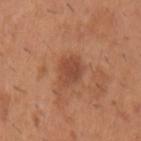Captured during whole-body skin photography for melanoma surveillance; the lesion was not biopsied.
A male subject aged 38 to 42.
The lesion is on the left upper arm.
Longest diameter approximately 3 mm.
A lesion tile, about 15 mm wide, cut from a 3D total-body photograph.
The total-body-photography lesion software estimated a lesion–skin lightness drop of about 9 and a lesion-to-skin contrast of about 6.5 (normalized; higher = more distinct). The software also gave border irregularity of about 2 on a 0–10 scale, a within-lesion color-variation index near 2/10, and peripheral color asymmetry of about 0.5. And it measured a classifier nevus-likeness of about 45/100 and a detector confidence of about 100 out of 100 that the crop contains a lesion.
This is a white-light tile.